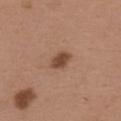Captured during whole-body skin photography for melanoma surveillance; the lesion was not biopsied. This is a white-light tile. A male patient aged around 40. Cropped from a total-body skin-imaging series; the visible field is about 15 mm. Located on the back.